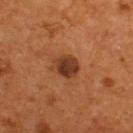Q: Is there a histopathology result?
A: no biopsy performed (imaged during a skin exam)
Q: Patient demographics?
A: male, aged around 55
Q: What lighting was used for the tile?
A: cross-polarized
Q: Lesion size?
A: ≈3 mm
Q: What did automated image analysis measure?
A: a footprint of about 6.5 mm², an eccentricity of roughly 0.55, and two-axis asymmetry of about 0.15; an average lesion color of about L≈34 a*≈23 b*≈31 (CIELAB), a lesion–skin lightness drop of about 12, and a lesion-to-skin contrast of about 10.5 (normalized; higher = more distinct); a border-irregularity rating of about 1.5/10, internal color variation of about 3.5 on a 0–10 scale, and a peripheral color-asymmetry measure near 1
Q: How was this image acquired?
A: ~15 mm crop, total-body skin-cancer survey
Q: Lesion location?
A: the upper back